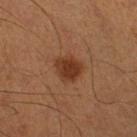Q: Was a biopsy performed?
A: imaged on a skin check; not biopsied
Q: Illumination type?
A: cross-polarized
Q: What did automated image analysis measure?
A: roughly 10 lightness units darker than nearby skin; a classifier nevus-likeness of about 95/100 and a detector confidence of about 100 out of 100 that the crop contains a lesion
Q: Who is the patient?
A: male, aged 48 to 52
Q: How large is the lesion?
A: about 3 mm
Q: How was this image acquired?
A: 15 mm crop, total-body photography
Q: Where on the body is the lesion?
A: the right lower leg